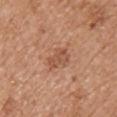Clinical impression:
Recorded during total-body skin imaging; not selected for excision or biopsy.
Image and clinical context:
The lesion is on the chest. Cropped from a total-body skin-imaging series; the visible field is about 15 mm. The patient is a female aged around 35. The recorded lesion diameter is about 3.5 mm. Automated image analysis of the tile measured a mean CIELAB color near L≈53 a*≈23 b*≈33 and roughly 9 lightness units darker than nearby skin. The software also gave a classifier nevus-likeness of about 5/100 and a lesion-detection confidence of about 100/100.